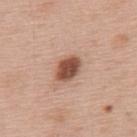Recorded during total-body skin imaging; not selected for excision or biopsy. The tile uses white-light illumination. A 15 mm close-up extracted from a 3D total-body photography capture. Longest diameter approximately 3.5 mm. The patient is a male aged 58 to 62. Located on the back.